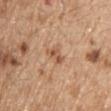notes: imaged on a skin check; not biopsied | image: ~15 mm crop, total-body skin-cancer survey | illumination: white-light illumination | subject: male, about 70 years old | site: the upper back.